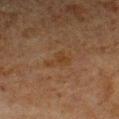| key | value |
|---|---|
| biopsy status | catalogued during a skin exam; not biopsied |
| image source | ~15 mm crop, total-body skin-cancer survey |
| subject | male, approximately 80 years of age |
| lighting | cross-polarized illumination |
| automated lesion analysis | a border-irregularity rating of about 5/10, a color-variation rating of about 2/10, and a peripheral color-asymmetry measure near 0.5; an automated nevus-likeness rating near 0 out of 100 |
| diameter | ~3.5 mm (longest diameter) |
| anatomic site | the left upper arm |